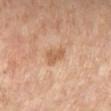Assessment:
Imaged during a routine full-body skin examination; the lesion was not biopsied and no histopathology is available.
Clinical summary:
From the left lower leg. Cropped from a total-body skin-imaging series; the visible field is about 15 mm. Imaged with cross-polarized lighting. A male patient aged 68–72.